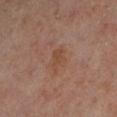{
  "biopsy_status": "not biopsied; imaged during a skin examination",
  "lesion_size": {
    "long_diameter_mm_approx": 3.0
  },
  "image": {
    "source": "total-body photography crop",
    "field_of_view_mm": 15
  },
  "lighting": "cross-polarized",
  "site": "left lower leg",
  "automated_metrics": {
    "cielab_L": 45,
    "cielab_a": 20,
    "cielab_b": 29,
    "vs_skin_contrast_norm": 5.5
  },
  "patient": {
    "sex": "male",
    "age_approx": 50
  }
}